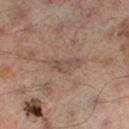imaging modality = 15 mm crop, total-body photography | tile lighting = cross-polarized illumination | location = the leg | subject = male, aged 63–67.